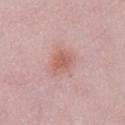The lesion was tiled from a total-body skin photograph and was not biopsied. A female subject, about 25 years old. The recorded lesion diameter is about 3.5 mm. A lesion tile, about 15 mm wide, cut from a 3D total-body photograph. The tile uses white-light illumination. On the front of the torso.